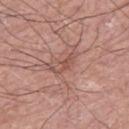The lesion was tiled from a total-body skin photograph and was not biopsied.
The lesion is on the right thigh.
This is a white-light tile.
The total-body-photography lesion software estimated a footprint of about 4 mm² and a shape-asymmetry score of about 0.45 (0 = symmetric). The software also gave about 8 CIELAB-L* units darker than the surrounding skin and a normalized lesion–skin contrast near 5.5. And it measured a color-variation rating of about 2/10 and peripheral color asymmetry of about 0.5.
The subject is a male aged 73–77.
A lesion tile, about 15 mm wide, cut from a 3D total-body photograph.